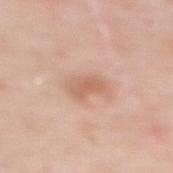Assessment: The lesion was photographed on a routine skin check and not biopsied; there is no pathology result. Background: A female subject, aged 48–52. The lesion-visualizer software estimated a footprint of about 5.5 mm², an eccentricity of roughly 0.8, and a symmetry-axis asymmetry near 0.3. The analysis additionally found internal color variation of about 2.5 on a 0–10 scale and a peripheral color-asymmetry measure near 1. And it measured an automated nevus-likeness rating near 60 out of 100 and lesion-presence confidence of about 100/100. The recorded lesion diameter is about 3 mm. Imaged with white-light lighting. Located on the upper back. Cropped from a total-body skin-imaging series; the visible field is about 15 mm.